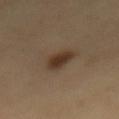| field | value |
|---|---|
| body site | the mid back |
| patient | female, aged around 60 |
| acquisition | total-body-photography crop, ~15 mm field of view |